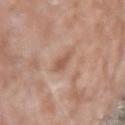Q: Was this lesion biopsied?
A: total-body-photography surveillance lesion; no biopsy
Q: How was this image acquired?
A: ~15 mm tile from a whole-body skin photo
Q: Lesion location?
A: the right upper arm
Q: What are the patient's age and sex?
A: male, aged 73 to 77
Q: Illumination type?
A: white-light illumination